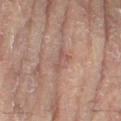The lesion was photographed on a routine skin check and not biopsied; there is no pathology result.
This image is a 15 mm lesion crop taken from a total-body photograph.
A female patient in their 80s.
Located on the left leg.